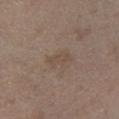Clinical impression:
Recorded during total-body skin imaging; not selected for excision or biopsy.
Context:
This is a white-light tile. About 3 mm across. Automated tile analysis of the lesion measured an average lesion color of about L≈47 a*≈13 b*≈24 (CIELAB), roughly 5 lightness units darker than nearby skin, and a lesion-to-skin contrast of about 4.5 (normalized; higher = more distinct). A female subject aged 63 to 67. Cropped from a whole-body photographic skin survey; the tile spans about 15 mm. The lesion is located on the leg.